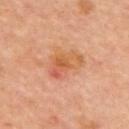Impression: Imaged during a routine full-body skin examination; the lesion was not biopsied and no histopathology is available. Clinical summary: A lesion tile, about 15 mm wide, cut from a 3D total-body photograph. A male patient aged approximately 60. From the upper back.